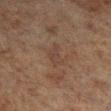follow-up: imaged on a skin check; not biopsied
location: the right lower leg
illumination: cross-polarized illumination
lesion diameter: ≈3.5 mm
subject: male, roughly 70 years of age
acquisition: 15 mm crop, total-body photography